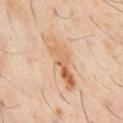{"biopsy_status": "not biopsied; imaged during a skin examination", "lighting": "cross-polarized", "patient": {"sex": "male", "age_approx": 60}, "image": {"source": "total-body photography crop", "field_of_view_mm": 15}, "site": "chest", "lesion_size": {"long_diameter_mm_approx": 7.5}}